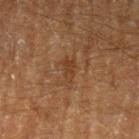Assessment: Captured during whole-body skin photography for melanoma surveillance; the lesion was not biopsied. Acquisition and patient details: A roughly 15 mm field-of-view crop from a total-body skin photograph. The patient is a male roughly 65 years of age. On the left upper arm.